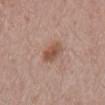Clinical impression:
Captured during whole-body skin photography for melanoma surveillance; the lesion was not biopsied.
Clinical summary:
A male patient, approximately 55 years of age. The lesion's longest dimension is about 3 mm. The lesion is located on the mid back. A region of skin cropped from a whole-body photographic capture, roughly 15 mm wide. Captured under white-light illumination.